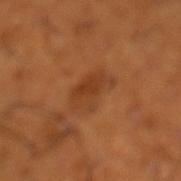Captured during whole-body skin photography for melanoma surveillance; the lesion was not biopsied. Captured under cross-polarized illumination. A male subject, roughly 65 years of age. A lesion tile, about 15 mm wide, cut from a 3D total-body photograph. Located on the left lower leg.